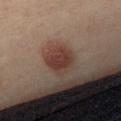About 3.5 mm across.
From the chest.
A 15 mm close-up tile from a total-body photography series done for melanoma screening.
Automated image analysis of the tile measured an area of roughly 9.5 mm², an outline eccentricity of about 0.3 (0 = round, 1 = elongated), and two-axis asymmetry of about 0.2. It also reported a border-irregularity index near 2/10 and internal color variation of about 3.5 on a 0–10 scale.
Imaged with white-light lighting.
A female patient, about 35 years old.